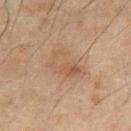{
  "biopsy_status": "not biopsied; imaged during a skin examination",
  "lesion_size": {
    "long_diameter_mm_approx": 4.5
  },
  "lighting": "cross-polarized",
  "site": "chest",
  "image": {
    "source": "total-body photography crop",
    "field_of_view_mm": 15
  },
  "patient": {
    "sex": "male",
    "age_approx": 70
  }
}